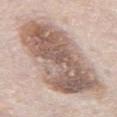The lesion was tiled from a total-body skin photograph and was not biopsied. The tile uses white-light illumination. Cropped from a total-body skin-imaging series; the visible field is about 15 mm. The lesion is located on the abdomen. A male subject in their mid- to late 70s. The total-body-photography lesion software estimated a border-irregularity index near 3/10, a within-lesion color-variation index near 8/10, and radial color variation of about 3. Approximately 12 mm at its widest.